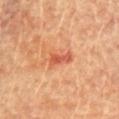Q: What is the imaging modality?
A: ~15 mm crop, total-body skin-cancer survey
Q: How large is the lesion?
A: ~3 mm (longest diameter)
Q: What lighting was used for the tile?
A: cross-polarized
Q: What is the anatomic site?
A: the front of the torso
Q: What did automated image analysis measure?
A: a border-irregularity index near 3.5/10, a within-lesion color-variation index near 1/10, and peripheral color asymmetry of about 0
Q: Patient demographics?
A: female, approximately 80 years of age